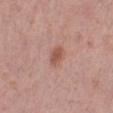Impression:
The lesion was photographed on a routine skin check and not biopsied; there is no pathology result.
Acquisition and patient details:
A female subject, about 40 years old. A 15 mm close-up extracted from a 3D total-body photography capture. Captured under white-light illumination. The total-body-photography lesion software estimated a footprint of about 4.5 mm², a shape eccentricity near 0.7, and a shape-asymmetry score of about 0.2 (0 = symmetric). It also reported a mean CIELAB color near L≈54 a*≈23 b*≈28, about 9 CIELAB-L* units darker than the surrounding skin, and a normalized border contrast of about 7. The software also gave border irregularity of about 1.5 on a 0–10 scale and radial color variation of about 1. On the leg.